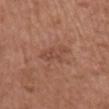Q: Automated lesion metrics?
A: a footprint of about 6 mm², an eccentricity of roughly 0.85, and a shape-asymmetry score of about 0.45 (0 = symmetric); roughly 7 lightness units darker than nearby skin and a normalized border contrast of about 5; a border-irregularity rating of about 5/10, a color-variation rating of about 2/10, and a peripheral color-asymmetry measure near 1; a classifier nevus-likeness of about 0/100 and a detector confidence of about 100 out of 100 that the crop contains a lesion
Q: What kind of image is this?
A: total-body-photography crop, ~15 mm field of view
Q: How large is the lesion?
A: about 4 mm
Q: Patient demographics?
A: female, aged 73 to 77
Q: Where on the body is the lesion?
A: the front of the torso